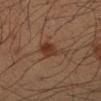Case summary:
* tile lighting: cross-polarized illumination
* patient: male, aged 33–37
* body site: the left forearm
* image source: ~15 mm crop, total-body skin-cancer survey